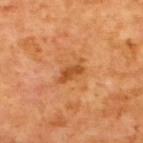Q: Is there a histopathology result?
A: imaged on a skin check; not biopsied
Q: Illumination type?
A: cross-polarized
Q: What did automated image analysis measure?
A: a footprint of about 4.5 mm² and a shape-asymmetry score of about 0.4 (0 = symmetric); a mean CIELAB color near L≈50 a*≈28 b*≈43, about 10 CIELAB-L* units darker than the surrounding skin, and a lesion-to-skin contrast of about 7 (normalized; higher = more distinct); border irregularity of about 4.5 on a 0–10 scale and a within-lesion color-variation index near 2/10
Q: Where on the body is the lesion?
A: the upper back
Q: What are the patient's age and sex?
A: male, in their mid- to late 60s
Q: How large is the lesion?
A: ≈3.5 mm
Q: What is the imaging modality?
A: 15 mm crop, total-body photography